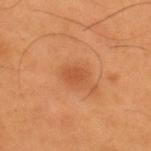Part of a total-body skin-imaging series; this lesion was reviewed on a skin check and was not flagged for biopsy.
A 15 mm close-up extracted from a 3D total-body photography capture.
About 2.5 mm across.
Located on the upper back.
The subject is a male aged 53–57.